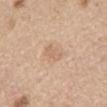biopsy status = catalogued during a skin exam; not biopsied
lighting = white-light
automated lesion analysis = a lesion area of about 4 mm², an eccentricity of roughly 0.9, and a symmetry-axis asymmetry near 0.25; a nevus-likeness score of about 5/100
subject = male, approximately 70 years of age
imaging modality = ~15 mm crop, total-body skin-cancer survey
location = the abdomen
size = ≈3 mm